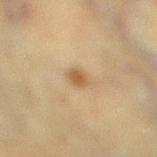No biopsy was performed on this lesion — it was imaged during a full skin examination and was not determined to be concerning. A female patient, roughly 55 years of age. The recorded lesion diameter is about 3 mm. Cropped from a whole-body photographic skin survey; the tile spans about 15 mm. The lesion is on the leg. This is a cross-polarized tile.